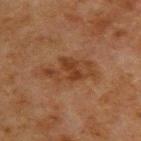Captured during whole-body skin photography for melanoma surveillance; the lesion was not biopsied. A male patient approximately 50 years of age. A 15 mm crop from a total-body photograph taken for skin-cancer surveillance. Automated image analysis of the tile measured an area of roughly 12 mm², a shape eccentricity near 0.9, and two-axis asymmetry of about 0.3. The software also gave a border-irregularity index near 5/10, internal color variation of about 4.5 on a 0–10 scale, and peripheral color asymmetry of about 1.5. It also reported a lesion-detection confidence of about 100/100. Captured under cross-polarized illumination. Measured at roughly 6 mm in maximum diameter. Located on the upper back.